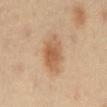| field | value |
|---|---|
| illumination | cross-polarized illumination |
| body site | the abdomen |
| patient | male, about 40 years old |
| acquisition | ~15 mm tile from a whole-body skin photo |